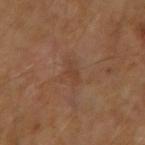The lesion was tiled from a total-body skin photograph and was not biopsied. The subject is aged approximately 55. The lesion-visualizer software estimated a lesion–skin lightness drop of about 5. It also reported a border-irregularity rating of about 4/10 and a peripheral color-asymmetry measure near 0. And it measured an automated nevus-likeness rating near 0 out of 100. A 15 mm close-up extracted from a 3D total-body photography capture. The lesion's longest dimension is about 2.5 mm. The lesion is on the right upper arm. The tile uses cross-polarized illumination.